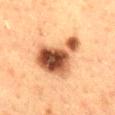follow-up: total-body-photography surveillance lesion; no biopsy
lesion diameter: about 6.5 mm
illumination: cross-polarized
automated metrics: a border-irregularity index near 5/10, a color-variation rating of about 10/10, and radial color variation of about 3; a nevus-likeness score of about 65/100
image: 15 mm crop, total-body photography
subject: female, aged around 55
body site: the mid back